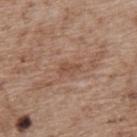Notes:
– workup — catalogued during a skin exam; not biopsied